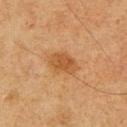Clinical impression:
The lesion was tiled from a total-body skin photograph and was not biopsied.
Context:
This is a cross-polarized tile. A roughly 15 mm field-of-view crop from a total-body skin photograph. The patient is a male aged 58–62. The lesion is located on the left upper arm.